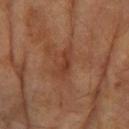biopsy_status: not biopsied; imaged during a skin examination
site: left forearm
automated_metrics:
  area_mm2_approx: 5.0
  eccentricity: 0.9
  shape_asymmetry: 0.4
  cielab_L: 35
  cielab_a: 22
  cielab_b: 28
  vs_skin_darker_L: 6.0
lesion_size:
  long_diameter_mm_approx: 4.0
patient:
  sex: female
  age_approx: 60
image:
  source: total-body photography crop
  field_of_view_mm: 15